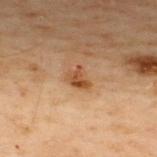Impression: Captured during whole-body skin photography for melanoma surveillance; the lesion was not biopsied. Context: The lesion is on the upper back. A 15 mm close-up extracted from a 3D total-body photography capture. The lesion's longest dimension is about 2.5 mm. Automated tile analysis of the lesion measured an average lesion color of about L≈41 a*≈20 b*≈31 (CIELAB) and roughly 9 lightness units darker than nearby skin. The analysis additionally found a nevus-likeness score of about 30/100. The tile uses cross-polarized illumination. A male patient, roughly 50 years of age.